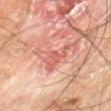biopsy status: total-body-photography surveillance lesion; no biopsy
illumination: cross-polarized illumination
lesion diameter: ~5 mm (longest diameter)
image: total-body-photography crop, ~15 mm field of view
site: the left thigh
subject: male, aged 68 to 72